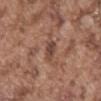Part of a total-body skin-imaging series; this lesion was reviewed on a skin check and was not flagged for biopsy. A 15 mm close-up tile from a total-body photography series done for melanoma screening. An algorithmic analysis of the crop reported a footprint of about 3.5 mm², a shape eccentricity near 0.85, and a symmetry-axis asymmetry near 0.25. It also reported a mean CIELAB color near L≈44 a*≈20 b*≈26 and roughly 10 lightness units darker than nearby skin. The analysis additionally found a border-irregularity rating of about 2.5/10, internal color variation of about 3.5 on a 0–10 scale, and radial color variation of about 1. It also reported a nevus-likeness score of about 0/100 and lesion-presence confidence of about 95/100. A male patient, approximately 75 years of age. Captured under white-light illumination. About 3 mm across. From the abdomen.